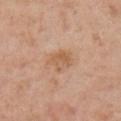workup = no biopsy performed (imaged during a skin exam)
image source = ~15 mm tile from a whole-body skin photo
site = the right upper arm
subject = male, aged 53 to 57